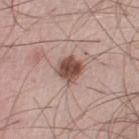Recorded during total-body skin imaging; not selected for excision or biopsy. A region of skin cropped from a whole-body photographic capture, roughly 15 mm wide. The lesion's longest dimension is about 3 mm. The lesion is on the right thigh. A male patient approximately 50 years of age.